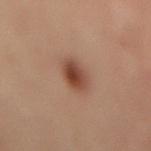biopsy status=catalogued during a skin exam; not biopsied
imaging modality=total-body-photography crop, ~15 mm field of view
anatomic site=the mid back
tile lighting=cross-polarized
subject=male, roughly 40 years of age
lesion size=~3.5 mm (longest diameter)
image-analysis metrics=a lesion area of about 6.5 mm²; about 10 CIELAB-L* units darker than the surrounding skin and a normalized lesion–skin contrast near 9.5